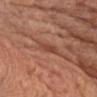The lesion was tiled from a total-body skin photograph and was not biopsied. A female subject, aged approximately 75. Imaged with white-light lighting. A roughly 15 mm field-of-view crop from a total-body skin photograph. On the chest. The lesion's longest dimension is about 3 mm. The total-body-photography lesion software estimated about 8 CIELAB-L* units darker than the surrounding skin and a lesion-to-skin contrast of about 6 (normalized; higher = more distinct).